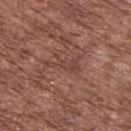The recorded lesion diameter is about 4 mm. The patient is a male about 75 years old. On the upper back. Imaged with white-light lighting. Cropped from a total-body skin-imaging series; the visible field is about 15 mm.